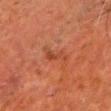{
  "biopsy_status": "not biopsied; imaged during a skin examination",
  "image": {
    "source": "total-body photography crop",
    "field_of_view_mm": 15
  },
  "site": "front of the torso",
  "automated_metrics": {
    "area_mm2_approx": 4.0,
    "shape_asymmetry": 0.65,
    "border_irregularity_0_10": 7.0,
    "peripheral_color_asymmetry": 0.0
  },
  "patient": {
    "sex": "male",
    "age_approx": 65
  }
}